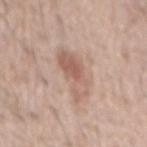Findings:
– follow-up · no biopsy performed (imaged during a skin exam)
– subject · male, aged around 65
– location · the mid back
– illumination · white-light illumination
– image · total-body-photography crop, ~15 mm field of view
– diameter · about 6 mm